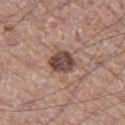| feature | finding |
|---|---|
| biopsy status | no biopsy performed (imaged during a skin exam) |
| automated metrics | two-axis asymmetry of about 0.1; an average lesion color of about L≈45 a*≈19 b*≈22 (CIELAB), a lesion–skin lightness drop of about 14, and a lesion-to-skin contrast of about 10.5 (normalized; higher = more distinct) |
| image source | 15 mm crop, total-body photography |
| patient | male, aged 63 to 67 |
| location | the left thigh |
| size | ≈3.5 mm |